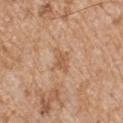This lesion was catalogued during total-body skin photography and was not selected for biopsy. Automated image analysis of the tile measured a footprint of about 4 mm², a shape eccentricity near 0.8, and a symmetry-axis asymmetry near 0.3. And it measured a lesion–skin lightness drop of about 8 and a lesion-to-skin contrast of about 6 (normalized; higher = more distinct). It also reported a nevus-likeness score of about 0/100 and lesion-presence confidence of about 100/100. A 15 mm close-up extracted from a 3D total-body photography capture. Captured under white-light illumination. A male subject, aged around 65. The lesion's longest dimension is about 3 mm. Located on the left upper arm.